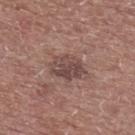Clinical impression: This lesion was catalogued during total-body skin photography and was not selected for biopsy. Context: The lesion is on the upper back. A male patient in their 60s. A 15 mm crop from a total-body photograph taken for skin-cancer surveillance.